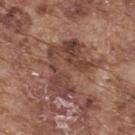Background:
A roughly 15 mm field-of-view crop from a total-body skin photograph. Located on the upper back. This is a white-light tile. A male subject, aged 73–77.